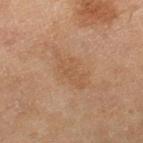<tbp_lesion>
<biopsy_status>not biopsied; imaged during a skin examination</biopsy_status>
<site>right lower leg</site>
<lesion_size>
  <long_diameter_mm_approx>5.5</long_diameter_mm_approx>
</lesion_size>
<lighting>cross-polarized</lighting>
<patient>
  <sex>female</sex>
  <age_approx>60</age_approx>
</patient>
<image>
  <source>total-body photography crop</source>
  <field_of_view_mm>15</field_of_view_mm>
</image>
</tbp_lesion>